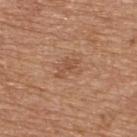Case summary:
- follow-up: imaged on a skin check; not biopsied
- tile lighting: white-light
- imaging modality: ~15 mm tile from a whole-body skin photo
- patient: male, roughly 65 years of age
- body site: the upper back